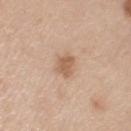Case summary:
- biopsy status · imaged on a skin check; not biopsied
- patient · male, in their 70s
- anatomic site · the left upper arm
- size · ~3 mm (longest diameter)
- image source · total-body-photography crop, ~15 mm field of view
- automated lesion analysis · a footprint of about 5 mm², an outline eccentricity of about 0.6 (0 = round, 1 = elongated), and a shape-asymmetry score of about 0.25 (0 = symmetric); border irregularity of about 2.5 on a 0–10 scale and radial color variation of about 0.5